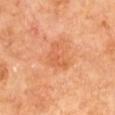biopsy_status: not biopsied; imaged during a skin examination
image:
  source: total-body photography crop
  field_of_view_mm: 15
patient:
  sex: male
  age_approx: 65
site: left upper arm
automated_metrics:
  cielab_L: 58
  cielab_a: 29
  cielab_b: 39
  vs_skin_darker_L: 7.0
  vs_skin_contrast_norm: 5.0
  peripheral_color_asymmetry: 0.5
  lesion_detection_confidence_0_100: 100